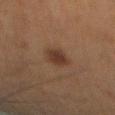Case summary:
– notes: catalogued during a skin exam; not biopsied
– lesion size: about 3.5 mm
– site: the mid back
– patient: male, aged 63–67
– acquisition: total-body-photography crop, ~15 mm field of view